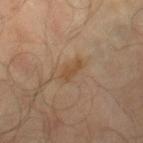Part of a total-body skin-imaging series; this lesion was reviewed on a skin check and was not flagged for biopsy. On the leg. This image is a 15 mm lesion crop taken from a total-body photograph. An algorithmic analysis of the crop reported a lesion color around L≈44 a*≈17 b*≈31 in CIELAB, about 7 CIELAB-L* units darker than the surrounding skin, and a normalized border contrast of about 6.5. The software also gave a classifier nevus-likeness of about 0/100. The lesion's longest dimension is about 2.5 mm. The patient is a male about 65 years old.